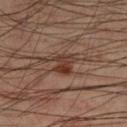Part of a total-body skin-imaging series; this lesion was reviewed on a skin check and was not flagged for biopsy. The lesion is on the leg. The subject is a male aged 43–47. This is a cross-polarized tile. Longest diameter approximately 2.5 mm. A region of skin cropped from a whole-body photographic capture, roughly 15 mm wide. An algorithmic analysis of the crop reported a lesion area of about 5 mm² and a shape eccentricity near 0.55. It also reported a lesion color around L≈34 a*≈19 b*≈24 in CIELAB, about 9 CIELAB-L* units darker than the surrounding skin, and a normalized border contrast of about 9.